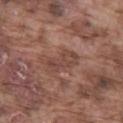follow-up = catalogued during a skin exam; not biopsied | anatomic site = the left thigh | tile lighting = white-light illumination | diameter = about 4.5 mm | TBP lesion metrics = a border-irregularity index near 3.5/10 and a within-lesion color-variation index near 3/10 | image = total-body-photography crop, ~15 mm field of view | patient = male, aged around 75.